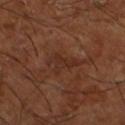Part of a total-body skin-imaging series; this lesion was reviewed on a skin check and was not flagged for biopsy. A male patient aged 63–67. A close-up tile cropped from a whole-body skin photograph, about 15 mm across. Located on the left lower leg.